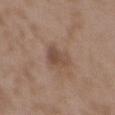workup: no biopsy performed (imaged during a skin exam)
patient: female, aged 33 to 37
lesion diameter: ≈3.5 mm
anatomic site: the chest
TBP lesion metrics: a footprint of about 6 mm², an eccentricity of roughly 0.8, and two-axis asymmetry of about 0.35; a nevus-likeness score of about 10/100 and a detector confidence of about 100 out of 100 that the crop contains a lesion
acquisition: ~15 mm tile from a whole-body skin photo
lighting: white-light illumination